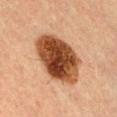biopsy_status: not biopsied; imaged during a skin examination
lesion_size:
  long_diameter_mm_approx: 7.5
image:
  source: total-body photography crop
  field_of_view_mm: 15
patient:
  sex: male
  age_approx: 35
site: chest
lighting: cross-polarized